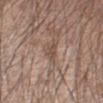The lesion was photographed on a routine skin check and not biopsied; there is no pathology result.
A region of skin cropped from a whole-body photographic capture, roughly 15 mm wide.
The tile uses white-light illumination.
Automated image analysis of the tile measured an area of roughly 3 mm², a shape eccentricity near 0.8, and two-axis asymmetry of about 0.4. And it measured an average lesion color of about L≈49 a*≈16 b*≈25 (CIELAB) and about 8 CIELAB-L* units darker than the surrounding skin. The analysis additionally found a border-irregularity rating of about 3.5/10, internal color variation of about 1 on a 0–10 scale, and peripheral color asymmetry of about 0.5. And it measured a nevus-likeness score of about 0/100 and a detector confidence of about 70 out of 100 that the crop contains a lesion.
A male subject aged approximately 45.
From the right forearm.
Longest diameter approximately 2.5 mm.